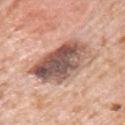{
  "biopsy_status": "not biopsied; imaged during a skin examination",
  "site": "arm",
  "patient": {
    "sex": "male",
    "age_approx": 80
  },
  "image": {
    "source": "total-body photography crop",
    "field_of_view_mm": 15
  }
}